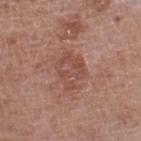Q: Is there a histopathology result?
A: no biopsy performed (imaged during a skin exam)
Q: What is the lesion's diameter?
A: ~4.5 mm (longest diameter)
Q: Where on the body is the lesion?
A: the right lower leg
Q: What kind of image is this?
A: ~15 mm tile from a whole-body skin photo
Q: How was the tile lit?
A: white-light illumination
Q: What are the patient's age and sex?
A: female, about 70 years old